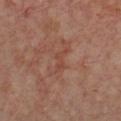| key | value |
|---|---|
| workup | catalogued during a skin exam; not biopsied |
| image | ~15 mm crop, total-body skin-cancer survey |
| site | the chest |
| diameter | ≈3 mm |
| lighting | cross-polarized illumination |
| patient | in their mid- to late 50s |